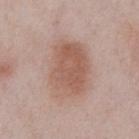Recorded during total-body skin imaging; not selected for excision or biopsy.
Located on the chest.
A male patient approximately 55 years of age.
Automated image analysis of the tile measured a lesion color around L≈57 a*≈20 b*≈26 in CIELAB, about 10 CIELAB-L* units darker than the surrounding skin, and a lesion-to-skin contrast of about 7 (normalized; higher = more distinct). The analysis additionally found border irregularity of about 2 on a 0–10 scale and internal color variation of about 4 on a 0–10 scale.
Cropped from a whole-body photographic skin survey; the tile spans about 15 mm.
This is a white-light tile.
Measured at roughly 6.5 mm in maximum diameter.